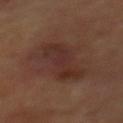biopsy_status: not biopsied; imaged during a skin examination
site: mid back
lesion_size:
  long_diameter_mm_approx: 7.0
image:
  source: total-body photography crop
  field_of_view_mm: 15
lighting: cross-polarized
patient:
  sex: male
  age_approx: 70
automated_metrics:
  cielab_L: 30
  cielab_a: 18
  cielab_b: 22
  vs_skin_darker_L: 6.0
  vs_skin_contrast_norm: 6.5